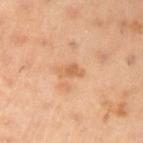| feature | finding |
|---|---|
| workup | no biopsy performed (imaged during a skin exam) |
| site | the left upper arm |
| imaging modality | ~15 mm tile from a whole-body skin photo |
| lighting | cross-polarized illumination |
| size | ~3 mm (longest diameter) |
| patient | male, aged 53–57 |